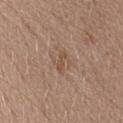Case summary:
- notes — catalogued during a skin exam; not biopsied
- subject — female, aged around 25
- lighting — white-light
- image source — ~15 mm tile from a whole-body skin photo
- body site — the head or neck